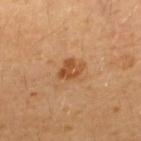follow-up: catalogued during a skin exam; not biopsied
diameter: ~3 mm (longest diameter)
image: ~15 mm tile from a whole-body skin photo
anatomic site: the back
tile lighting: cross-polarized
subject: male, roughly 30 years of age